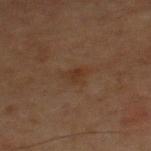The lesion was tiled from a total-body skin photograph and was not biopsied. Approximately 3 mm at its widest. The patient is a male aged 48–52. A lesion tile, about 15 mm wide, cut from a 3D total-body photograph. The lesion is located on the chest. Automated tile analysis of the lesion measured a lesion area of about 4.5 mm², an outline eccentricity of about 0.8 (0 = round, 1 = elongated), and a shape-asymmetry score of about 0.4 (0 = symmetric). The software also gave a nevus-likeness score of about 0/100 and lesion-presence confidence of about 100/100. This is a cross-polarized tile.